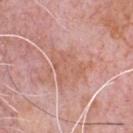<lesion>
  <image>
    <source>total-body photography crop</source>
    <field_of_view_mm>15</field_of_view_mm>
  </image>
  <patient>
    <sex>male</sex>
    <age_approx>80</age_approx>
  </patient>
  <site>chest</site>
</lesion>